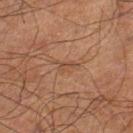No biopsy was performed on this lesion — it was imaged during a full skin examination and was not determined to be concerning. A close-up tile cropped from a whole-body skin photograph, about 15 mm across. A male subject aged 68–72. The total-body-photography lesion software estimated a lesion area of about 3 mm², a shape eccentricity near 0.9, and a symmetry-axis asymmetry near 0.55. The software also gave an average lesion color of about L≈38 a*≈17 b*≈26 (CIELAB), a lesion–skin lightness drop of about 5, and a lesion-to-skin contrast of about 5 (normalized; higher = more distinct). The analysis additionally found a border-irregularity index near 6/10, internal color variation of about 0 on a 0–10 scale, and a peripheral color-asymmetry measure near 0. The lesion is located on the left lower leg. The tile uses cross-polarized illumination.